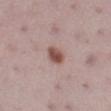<tbp_lesion>
<biopsy_status>not biopsied; imaged during a skin examination</biopsy_status>
<automated_metrics>
  <area_mm2_approx>4.5</area_mm2_approx>
  <eccentricity>0.8</eccentricity>
  <shape_asymmetry>0.2</shape_asymmetry>
</automated_metrics>
<image>
  <source>total-body photography crop</source>
  <field_of_view_mm>15</field_of_view_mm>
</image>
<patient>
  <sex>female</sex>
  <age_approx>35</age_approx>
</patient>
<site>right lower leg</site>
<lighting>white-light</lighting>
<lesion_size>
  <long_diameter_mm_approx>3.0</long_diameter_mm_approx>
</lesion_size>
</tbp_lesion>